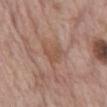Context: The tile uses white-light illumination. The recorded lesion diameter is about 2.5 mm. A male subject approximately 70 years of age. A 15 mm crop from a total-body photograph taken for skin-cancer surveillance. On the abdomen. The lesion-visualizer software estimated a mean CIELAB color near L≈52 a*≈20 b*≈29 and a lesion-to-skin contrast of about 5.5 (normalized; higher = more distinct). It also reported a nevus-likeness score of about 0/100.